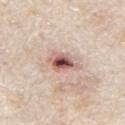Imaged during a routine full-body skin examination; the lesion was not biopsied and no histopathology is available.
Located on the mid back.
Automated tile analysis of the lesion measured a footprint of about 7.5 mm², an outline eccentricity of about 0.7 (0 = round, 1 = elongated), and two-axis asymmetry of about 0.3. And it measured an automated nevus-likeness rating near 0 out of 100.
Cropped from a whole-body photographic skin survey; the tile spans about 15 mm.
About 4 mm across.
The patient is a male in their mid- to late 60s.